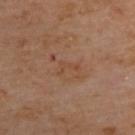| feature | finding |
|---|---|
| follow-up | catalogued during a skin exam; not biopsied |
| subject | female, about 60 years old |
| lesion size | ≈2.5 mm |
| imaging modality | total-body-photography crop, ~15 mm field of view |
| site | the upper back |
| tile lighting | cross-polarized illumination |